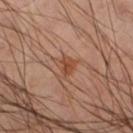Impression:
Captured during whole-body skin photography for melanoma surveillance; the lesion was not biopsied.
Context:
Automated image analysis of the tile measured an outline eccentricity of about 0.55 (0 = round, 1 = elongated) and a shape-asymmetry score of about 0.45 (0 = symmetric). And it measured a lesion-to-skin contrast of about 7.5 (normalized; higher = more distinct). And it measured lesion-presence confidence of about 100/100. The lesion is located on the leg. A close-up tile cropped from a whole-body skin photograph, about 15 mm across. A male subject in their 50s. Captured under cross-polarized illumination.